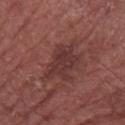Captured during whole-body skin photography for melanoma surveillance; the lesion was not biopsied. The patient is a male aged approximately 75. From the left forearm. An algorithmic analysis of the crop reported a lesion color around L≈35 a*≈23 b*≈20 in CIELAB, roughly 8 lightness units darker than nearby skin, and a normalized lesion–skin contrast near 7. It also reported border irregularity of about 4.5 on a 0–10 scale and a color-variation rating of about 2.5/10. The software also gave an automated nevus-likeness rating near 0 out of 100. Approximately 5.5 mm at its widest. Imaged with white-light lighting. Cropped from a total-body skin-imaging series; the visible field is about 15 mm.